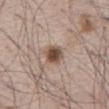• workup: catalogued during a skin exam; not biopsied
• acquisition: ~15 mm tile from a whole-body skin photo
• site: the chest
• patient: male, aged approximately 65